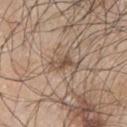Impression: The lesion was photographed on a routine skin check and not biopsied; there is no pathology result. Background: Longest diameter approximately 3.5 mm. A male patient in their 70s. The lesion is located on the left upper arm. The lesion-visualizer software estimated a lesion color around L≈52 a*≈15 b*≈27 in CIELAB and a lesion–skin lightness drop of about 10. A region of skin cropped from a whole-body photographic capture, roughly 15 mm wide.